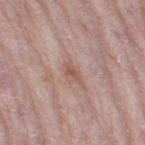notes: imaged on a skin check; not biopsied | subject: female, aged 68 to 72 | lighting: white-light illumination | automated lesion analysis: a footprint of about 2.5 mm², an outline eccentricity of about 0.85 (0 = round, 1 = elongated), and a shape-asymmetry score of about 0.3 (0 = symmetric); border irregularity of about 3 on a 0–10 scale, a color-variation rating of about 0/10, and radial color variation of about 0; an automated nevus-likeness rating near 0 out of 100 and a detector confidence of about 95 out of 100 that the crop contains a lesion | acquisition: total-body-photography crop, ~15 mm field of view | lesion size: about 2.5 mm | site: the right thigh.